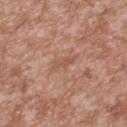image-analysis metrics: a lesion color around L≈54 a*≈21 b*≈30 in CIELAB and a normalized border contrast of about 5; a border-irregularity index near 5/10, internal color variation of about 0 on a 0–10 scale, and radial color variation of about 0; a nevus-likeness score of about 0/100 and a lesion-detection confidence of about 100/100
lesion size: ~3 mm (longest diameter)
tile lighting: white-light illumination
image: ~15 mm tile from a whole-body skin photo
anatomic site: the upper back
patient: male, in their mid- to late 40s